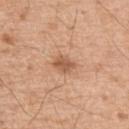tile lighting: white-light
anatomic site: the upper back
TBP lesion metrics: an area of roughly 4.5 mm² and an eccentricity of roughly 0.6; an automated nevus-likeness rating near 20 out of 100
lesion size: ~2.5 mm (longest diameter)
subject: male, aged 53 to 57
imaging modality: ~15 mm crop, total-body skin-cancer survey